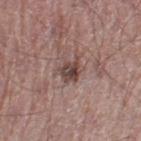Imaged during a routine full-body skin examination; the lesion was not biopsied and no histopathology is available.
The lesion-visualizer software estimated a footprint of about 4.5 mm², an outline eccentricity of about 0.55 (0 = round, 1 = elongated), and two-axis asymmetry of about 0.4. The software also gave a nevus-likeness score of about 10/100 and a detector confidence of about 100 out of 100 that the crop contains a lesion.
A male subject in their 60s.
A 15 mm close-up extracted from a 3D total-body photography capture.
Located on the left thigh.
Measured at roughly 3 mm in maximum diameter.